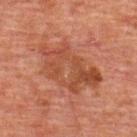The lesion is located on the upper back. A roughly 15 mm field-of-view crop from a total-body skin photograph. The subject is a male in their 60s. Measured at roughly 8 mm in maximum diameter.